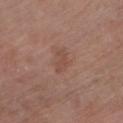| key | value |
|---|---|
| workup | catalogued during a skin exam; not biopsied |
| lighting | white-light |
| lesion size | about 3.5 mm |
| image source | ~15 mm crop, total-body skin-cancer survey |
| patient | male, approximately 60 years of age |
| location | the right upper arm |